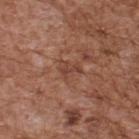The lesion was photographed on a routine skin check and not biopsied; there is no pathology result.